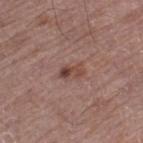<case>
  <biopsy_status>not biopsied; imaged during a skin examination</biopsy_status>
  <site>left thigh</site>
  <patient>
    <sex>male</sex>
    <age_approx>70</age_approx>
  </patient>
  <image>
    <source>total-body photography crop</source>
    <field_of_view_mm>15</field_of_view_mm>
  </image>
  <lighting>white-light</lighting>
</case>